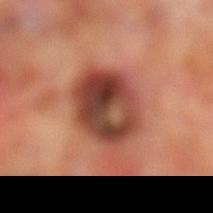{
  "biopsy_status": "not biopsied; imaged during a skin examination",
  "lighting": "cross-polarized",
  "image": {
    "source": "total-body photography crop",
    "field_of_view_mm": 15
  },
  "patient": {
    "sex": "male",
    "age_approx": 70
  },
  "site": "leg",
  "lesion_size": {
    "long_diameter_mm_approx": 6.0
  }
}